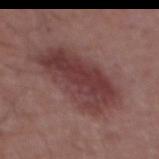{
  "biopsy_status": "not biopsied; imaged during a skin examination",
  "site": "abdomen",
  "lesion_size": {
    "long_diameter_mm_approx": 8.0
  },
  "image": {
    "source": "total-body photography crop",
    "field_of_view_mm": 15
  },
  "patient": {
    "sex": "male",
    "age_approx": 55
  }
}